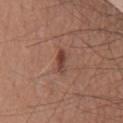biopsy status: catalogued during a skin exam; not biopsied
automated metrics: an area of roughly 3.5 mm², a shape eccentricity near 0.9, and two-axis asymmetry of about 0.3; a mean CIELAB color near L≈42 a*≈22 b*≈26, a lesion–skin lightness drop of about 10, and a normalized lesion–skin contrast near 8; a color-variation rating of about 2.5/10 and radial color variation of about 0.5; lesion-presence confidence of about 100/100
tile lighting: white-light illumination
subject: male, aged 53–57
lesion diameter: ~3 mm (longest diameter)
image: 15 mm crop, total-body photography